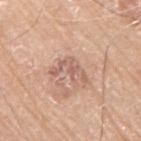The lesion was photographed on a routine skin check and not biopsied; there is no pathology result.
On the left arm.
A 15 mm close-up tile from a total-body photography series done for melanoma screening.
A male patient, aged approximately 80.